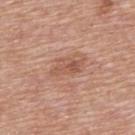Imaged during a routine full-body skin examination; the lesion was not biopsied and no histopathology is available. Captured under white-light illumination. Automated tile analysis of the lesion measured a footprint of about 5 mm², an outline eccentricity of about 0.9 (0 = round, 1 = elongated), and a symmetry-axis asymmetry near 0.3. It also reported a classifier nevus-likeness of about 0/100 and a detector confidence of about 100 out of 100 that the crop contains a lesion. Approximately 4 mm at its widest. From the front of the torso. A female patient, aged around 65. A roughly 15 mm field-of-view crop from a total-body skin photograph.